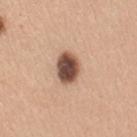follow-up=catalogued during a skin exam; not biopsied.